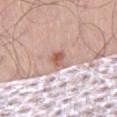Q: Was a biopsy performed?
A: total-body-photography surveillance lesion; no biopsy
Q: Who is the patient?
A: male, aged 68–72
Q: Illumination type?
A: white-light illumination
Q: What kind of image is this?
A: ~15 mm crop, total-body skin-cancer survey
Q: What is the anatomic site?
A: the right thigh
Q: What did automated image analysis measure?
A: a mean CIELAB color near L≈62 a*≈20 b*≈26, a lesion–skin lightness drop of about 11, and a normalized lesion–skin contrast near 7.5; a color-variation rating of about 7/10
Q: Lesion size?
A: ≈3 mm